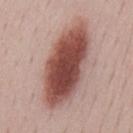Q: Was this lesion biopsied?
A: imaged on a skin check; not biopsied
Q: Who is the patient?
A: male, in their 50s
Q: What kind of image is this?
A: 15 mm crop, total-body photography
Q: Automated lesion metrics?
A: an area of roughly 38 mm², an eccentricity of roughly 0.9, and a shape-asymmetry score of about 0.1 (0 = symmetric); a within-lesion color-variation index near 7/10 and radial color variation of about 2; an automated nevus-likeness rating near 100 out of 100 and lesion-presence confidence of about 100/100
Q: How large is the lesion?
A: ~10.5 mm (longest diameter)
Q: Where on the body is the lesion?
A: the mid back